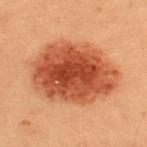workup = total-body-photography surveillance lesion; no biopsy
lesion size = ~8.5 mm (longest diameter)
subject = female, aged around 20
illumination = cross-polarized illumination
site = the upper back
image source = ~15 mm tile from a whole-body skin photo